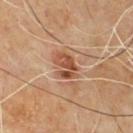{
  "biopsy_status": "not biopsied; imaged during a skin examination"
}